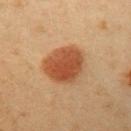Imaged during a routine full-body skin examination; the lesion was not biopsied and no histopathology is available. A male patient, approximately 40 years of age. An algorithmic analysis of the crop reported a lesion area of about 17 mm² and a symmetry-axis asymmetry near 0.15. The analysis additionally found an average lesion color of about L≈45 a*≈23 b*≈34 (CIELAB), roughly 12 lightness units darker than nearby skin, and a lesion-to-skin contrast of about 9.5 (normalized; higher = more distinct). Imaged with cross-polarized lighting. Located on the arm. A 15 mm close-up tile from a total-body photography series done for melanoma screening. The recorded lesion diameter is about 5 mm.